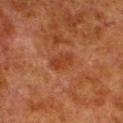follow-up=imaged on a skin check; not biopsied
anatomic site=the leg
diameter=≈3 mm
imaging modality=15 mm crop, total-body photography
patient=male, approximately 80 years of age
tile lighting=cross-polarized illumination